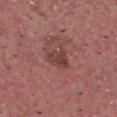<record>
<biopsy_status>not biopsied; imaged during a skin examination</biopsy_status>
<patient>
  <sex>male</sex>
  <age_approx>55</age_approx>
</patient>
<image>
  <source>total-body photography crop</source>
  <field_of_view_mm>15</field_of_view_mm>
</image>
<site>head or neck</site>
</record>